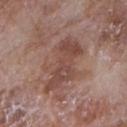Imaged during a routine full-body skin examination; the lesion was not biopsied and no histopathology is available.
From the right upper arm.
A 15 mm close-up extracted from a 3D total-body photography capture.
A female subject, aged 68 to 72.
Automated image analysis of the tile measured an area of roughly 17 mm², an eccentricity of roughly 0.85, and two-axis asymmetry of about 0.3. It also reported a normalized lesion–skin contrast near 7. It also reported a within-lesion color-variation index near 3.5/10 and radial color variation of about 1. The software also gave an automated nevus-likeness rating near 0 out of 100 and lesion-presence confidence of about 100/100.
Imaged with white-light lighting.